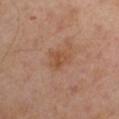Clinical impression: Recorded during total-body skin imaging; not selected for excision or biopsy. Background: A roughly 15 mm field-of-view crop from a total-body skin photograph. About 3 mm across. This is a cross-polarized tile. A male patient, approximately 55 years of age. An algorithmic analysis of the crop reported a footprint of about 5.5 mm², an outline eccentricity of about 0.5 (0 = round, 1 = elongated), and a shape-asymmetry score of about 0.35 (0 = symmetric). The analysis additionally found an average lesion color of about L≈50 a*≈21 b*≈32 (CIELAB), roughly 7 lightness units darker than nearby skin, and a lesion-to-skin contrast of about 6 (normalized; higher = more distinct). It also reported a classifier nevus-likeness of about 0/100 and a lesion-detection confidence of about 100/100. On the arm.